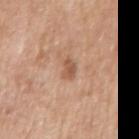notes: total-body-photography surveillance lesion; no biopsy
lighting: white-light
diameter: ≈2.5 mm
location: the right upper arm
subject: male, roughly 70 years of age
imaging modality: total-body-photography crop, ~15 mm field of view